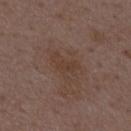Part of a total-body skin-imaging series; this lesion was reviewed on a skin check and was not flagged for biopsy.
Cropped from a total-body skin-imaging series; the visible field is about 15 mm.
From the back.
The recorded lesion diameter is about 3.5 mm.
Imaged with white-light lighting.
The total-body-photography lesion software estimated a symmetry-axis asymmetry near 0.5. The analysis additionally found a nevus-likeness score of about 0/100 and a lesion-detection confidence of about 100/100.
A male patient aged 48 to 52.